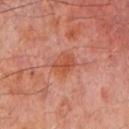Part of a total-body skin-imaging series; this lesion was reviewed on a skin check and was not flagged for biopsy.
A male subject aged 58 to 62.
Captured under cross-polarized illumination.
From the chest.
Cropped from a whole-body photographic skin survey; the tile spans about 15 mm.